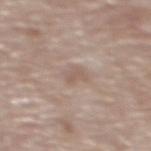Clinical impression: Imaged during a routine full-body skin examination; the lesion was not biopsied and no histopathology is available. Clinical summary: Approximately 2.5 mm at its widest. The lesion is located on the upper back. The subject is a male approximately 85 years of age or older. Cropped from a total-body skin-imaging series; the visible field is about 15 mm. Captured under white-light illumination. The lesion-visualizer software estimated an outline eccentricity of about 0.85 (0 = round, 1 = elongated) and a symmetry-axis asymmetry near 0.35. The software also gave a classifier nevus-likeness of about 0/100 and a lesion-detection confidence of about 100/100.